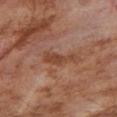A male patient approximately 55 years of age. The lesion is on the right upper arm. A lesion tile, about 15 mm wide, cut from a 3D total-body photograph.